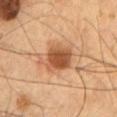The lesion was tiled from a total-body skin photograph and was not biopsied.
Automated image analysis of the tile measured an average lesion color of about L≈48 a*≈22 b*≈34 (CIELAB). The software also gave internal color variation of about 4.5 on a 0–10 scale and a peripheral color-asymmetry measure near 1.5. And it measured a nevus-likeness score of about 90/100 and a lesion-detection confidence of about 100/100.
A male patient about 55 years old.
The recorded lesion diameter is about 4.5 mm.
The lesion is on the chest.
A 15 mm close-up tile from a total-body photography series done for melanoma screening.
Captured under cross-polarized illumination.